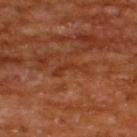Imaged during a routine full-body skin examination; the lesion was not biopsied and no histopathology is available.
A male patient in their mid-60s.
This is a cross-polarized tile.
A region of skin cropped from a whole-body photographic capture, roughly 15 mm wide.
On the upper back.